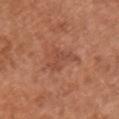Q: Lesion size?
A: ≈2.5 mm
Q: Illumination type?
A: white-light
Q: Who is the patient?
A: female, aged approximately 65
Q: How was this image acquired?
A: ~15 mm crop, total-body skin-cancer survey
Q: Where on the body is the lesion?
A: the chest